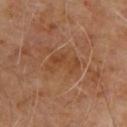Impression: Part of a total-body skin-imaging series; this lesion was reviewed on a skin check and was not flagged for biopsy. Context: Captured under cross-polarized illumination. Automated image analysis of the tile measured an area of roughly 7.5 mm², an eccentricity of roughly 0.85, and a shape-asymmetry score of about 0.4 (0 = symmetric). The analysis additionally found a border-irregularity index near 5/10, a color-variation rating of about 3/10, and radial color variation of about 1. The software also gave a classifier nevus-likeness of about 0/100 and lesion-presence confidence of about 100/100. A close-up tile cropped from a whole-body skin photograph, about 15 mm across. The lesion is located on the upper back. A male patient, approximately 65 years of age. About 4.5 mm across.